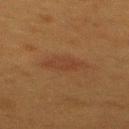follow-up = imaged on a skin check; not biopsied
patient = female, aged approximately 40
acquisition = ~15 mm crop, total-body skin-cancer survey
diameter = about 4.5 mm
body site = the upper back
automated metrics = a normalized border contrast of about 5; a border-irregularity index near 3/10, internal color variation of about 2 on a 0–10 scale, and peripheral color asymmetry of about 0.5; lesion-presence confidence of about 100/100